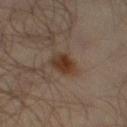Clinical impression:
Recorded during total-body skin imaging; not selected for excision or biopsy.
Background:
From the left thigh. A 15 mm close-up extracted from a 3D total-body photography capture. Automated tile analysis of the lesion measured a mean CIELAB color near L≈33 a*≈15 b*≈25, roughly 9 lightness units darker than nearby skin, and a normalized border contrast of about 9.5. And it measured a border-irregularity index near 2.5/10, internal color variation of about 4 on a 0–10 scale, and peripheral color asymmetry of about 1.5. It also reported a nevus-likeness score of about 95/100. A male subject aged 63–67.